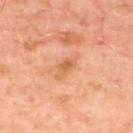biopsy status=imaged on a skin check; not biopsied | location=the upper back | acquisition=15 mm crop, total-body photography | TBP lesion metrics=a within-lesion color-variation index near 2/10 and radial color variation of about 0.5; a classifier nevus-likeness of about 0/100 and a lesion-detection confidence of about 100/100 | subject=male, roughly 50 years of age | size=~2.5 mm (longest diameter).